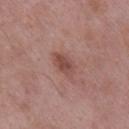{
  "biopsy_status": "not biopsied; imaged during a skin examination",
  "automated_metrics": {
    "area_mm2_approx": 5.5,
    "eccentricity": 0.8,
    "shape_asymmetry": 0.3,
    "cielab_L": 48,
    "cielab_a": 22,
    "cielab_b": 24,
    "border_irregularity_0_10": 3.0,
    "color_variation_0_10": 3.0,
    "peripheral_color_asymmetry": 1.0,
    "nevus_likeness_0_100": 30,
    "lesion_detection_confidence_0_100": 100
  },
  "patient": {
    "sex": "male",
    "age_approx": 55
  },
  "site": "leg",
  "image": {
    "source": "total-body photography crop",
    "field_of_view_mm": 15
  }
}